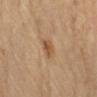{
  "biopsy_status": "not biopsied; imaged during a skin examination",
  "patient": {
    "sex": "female",
    "age_approx": 65
  },
  "site": "left forearm",
  "image": {
    "source": "total-body photography crop",
    "field_of_view_mm": 15
  }
}